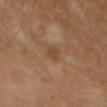Q: Was a biopsy performed?
A: imaged on a skin check; not biopsied
Q: Illumination type?
A: cross-polarized
Q: Patient demographics?
A: female, aged approximately 50
Q: How large is the lesion?
A: ≈2 mm
Q: What is the imaging modality?
A: total-body-photography crop, ~15 mm field of view
Q: What is the anatomic site?
A: the head or neck
Q: What did automated image analysis measure?
A: a lesion area of about 2.5 mm² and an eccentricity of roughly 0.45; a border-irregularity rating of about 2/10, internal color variation of about 2 on a 0–10 scale, and peripheral color asymmetry of about 1; lesion-presence confidence of about 100/100